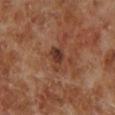Impression: This lesion was catalogued during total-body skin photography and was not selected for biopsy. Context: The lesion is on the leg. Longest diameter approximately 3 mm. Captured under cross-polarized illumination. A male subject, aged 68 to 72. A lesion tile, about 15 mm wide, cut from a 3D total-body photograph.